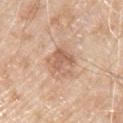Clinical impression: The lesion was tiled from a total-body skin photograph and was not biopsied. Context: This is a white-light tile. Automated image analysis of the tile measured an area of roughly 8 mm² and an eccentricity of roughly 0.45. The software also gave a mean CIELAB color near L≈61 a*≈20 b*≈31 and a lesion–skin lightness drop of about 10. And it measured a nevus-likeness score of about 0/100 and lesion-presence confidence of about 100/100. About 3.5 mm across. The lesion is located on the right upper arm. A male subject, in their 80s. A 15 mm close-up tile from a total-body photography series done for melanoma screening.